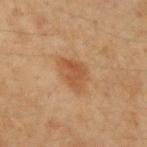{"biopsy_status": "not biopsied; imaged during a skin examination", "lighting": "cross-polarized", "image": {"source": "total-body photography crop", "field_of_view_mm": 15}, "site": "left forearm", "patient": {"sex": "female", "age_approx": 40}}